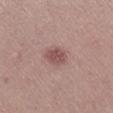follow-up = no biopsy performed (imaged during a skin exam).